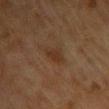{"biopsy_status": "not biopsied; imaged during a skin examination", "lesion_size": {"long_diameter_mm_approx": 3.0}, "image": {"source": "total-body photography crop", "field_of_view_mm": 15}, "lighting": "cross-polarized", "site": "chest", "patient": {"sex": "female", "age_approx": 80}}